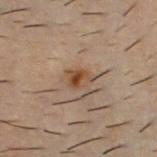Assessment:
Recorded during total-body skin imaging; not selected for excision or biopsy.
Clinical summary:
Captured under cross-polarized illumination. The lesion is located on the chest. A male subject aged approximately 30. A 15 mm close-up tile from a total-body photography series done for melanoma screening. Measured at roughly 2.5 mm in maximum diameter. Automated image analysis of the tile measured a border-irregularity index near 3/10 and a within-lesion color-variation index near 5.5/10.